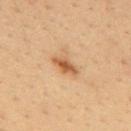| key | value |
|---|---|
| follow-up | catalogued during a skin exam; not biopsied |
| automated metrics | an area of roughly 4 mm² and a shape-asymmetry score of about 0.3 (0 = symmetric); a nevus-likeness score of about 85/100 and lesion-presence confidence of about 100/100 |
| lighting | cross-polarized |
| image | 15 mm crop, total-body photography |
| patient | male, approximately 35 years of age |
| site | the mid back |
| diameter | about 3.5 mm |